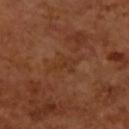{"biopsy_status": "not biopsied; imaged during a skin examination"}